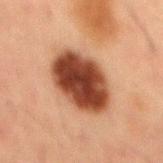Clinical impression: This lesion was catalogued during total-body skin photography and was not selected for biopsy. Acquisition and patient details: A male subject, aged 48–52. The recorded lesion diameter is about 7 mm. The lesion-visualizer software estimated a lesion color around L≈34 a*≈21 b*≈27 in CIELAB and roughly 18 lightness units darker than nearby skin. And it measured a border-irregularity index near 1/10, internal color variation of about 6 on a 0–10 scale, and a peripheral color-asymmetry measure near 2. The software also gave a detector confidence of about 100 out of 100 that the crop contains a lesion. The lesion is on the back. This is a cross-polarized tile. A 15 mm close-up tile from a total-body photography series done for melanoma screening.